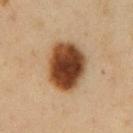{"biopsy_status": "not biopsied; imaged during a skin examination", "lesion_size": {"long_diameter_mm_approx": 6.0}, "site": "front of the torso", "patient": {"sex": "male", "age_approx": 50}, "image": {"source": "total-body photography crop", "field_of_view_mm": 15}}